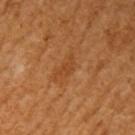{
  "biopsy_status": "not biopsied; imaged during a skin examination",
  "site": "arm",
  "lighting": "cross-polarized",
  "automated_metrics": {
    "area_mm2_approx": 3.5,
    "shape_asymmetry": 0.45,
    "cielab_L": 45,
    "cielab_a": 25,
    "cielab_b": 40,
    "vs_skin_darker_L": 6.0,
    "vs_skin_contrast_norm": 5.0,
    "nevus_likeness_0_100": 0
  },
  "patient": {
    "sex": "female",
    "age_approx": 55
  },
  "image": {
    "source": "total-body photography crop",
    "field_of_view_mm": 15
  }
}